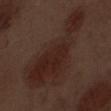Q: Was a biopsy performed?
A: imaged on a skin check; not biopsied
Q: Lesion size?
A: ≈12.5 mm
Q: What are the patient's age and sex?
A: male, approximately 70 years of age
Q: Where on the body is the lesion?
A: the front of the torso
Q: How was this image acquired?
A: total-body-photography crop, ~15 mm field of view
Q: What lighting was used for the tile?
A: white-light illumination